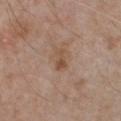Imaged during a routine full-body skin examination; the lesion was not biopsied and no histopathology is available. Located on the chest. Measured at roughly 2.5 mm in maximum diameter. Cropped from a whole-body photographic skin survey; the tile spans about 15 mm. Automated image analysis of the tile measured border irregularity of about 3.5 on a 0–10 scale, a color-variation rating of about 1.5/10, and radial color variation of about 0.5. And it measured a classifier nevus-likeness of about 0/100 and a lesion-detection confidence of about 100/100. The subject is a male in their mid-50s.